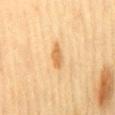{
  "image": {
    "source": "total-body photography crop",
    "field_of_view_mm": 15
  },
  "patient": {
    "sex": "female",
    "age_approx": 55
  },
  "lesion_size": {
    "long_diameter_mm_approx": 3.5
  },
  "automated_metrics": {
    "area_mm2_approx": 4.5,
    "eccentricity": 0.85,
    "shape_asymmetry": 0.2,
    "cielab_L": 57,
    "cielab_a": 18,
    "cielab_b": 39,
    "vs_skin_darker_L": 9.0,
    "vs_skin_contrast_norm": 7.0,
    "border_irregularity_0_10": 2.0,
    "color_variation_0_10": 2.0,
    "peripheral_color_asymmetry": 0.5
  },
  "site": "mid back"
}